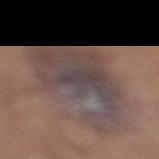notes = total-body-photography surveillance lesion; no biopsy